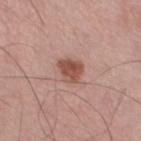biopsy status = no biopsy performed (imaged during a skin exam)
patient = male, aged approximately 70
automated lesion analysis = an area of roughly 6 mm², a shape eccentricity near 0.45, and two-axis asymmetry of about 0.3; an average lesion color of about L≈51 a*≈23 b*≈26 (CIELAB), about 12 CIELAB-L* units darker than the surrounding skin, and a normalized lesion–skin contrast near 9
lighting = white-light illumination
site = the right thigh
acquisition = ~15 mm tile from a whole-body skin photo